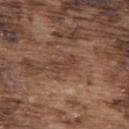| key | value |
|---|---|
| biopsy status | no biopsy performed (imaged during a skin exam) |
| image-analysis metrics | a lesion area of about 3.5 mm²; border irregularity of about 5.5 on a 0–10 scale and peripheral color asymmetry of about 0 |
| tile lighting | white-light illumination |
| patient | male, approximately 75 years of age |
| location | the upper back |
| image | ~15 mm tile from a whole-body skin photo |
| size | ≈3 mm |